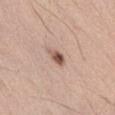Captured during whole-body skin photography for melanoma surveillance; the lesion was not biopsied.
A region of skin cropped from a whole-body photographic capture, roughly 15 mm wide.
About 2.5 mm across.
The patient is a male aged 53–57.
The tile uses white-light illumination.
An algorithmic analysis of the crop reported a lesion color around L≈54 a*≈20 b*≈26 in CIELAB, roughly 15 lightness units darker than nearby skin, and a normalized lesion–skin contrast near 10. The software also gave a border-irregularity rating of about 3.5/10, a color-variation rating of about 5/10, and peripheral color asymmetry of about 2. The software also gave lesion-presence confidence of about 100/100.
The lesion is located on the abdomen.